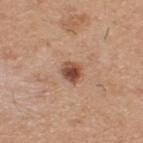{"automated_metrics": {"border_irregularity_0_10": 1.5, "color_variation_0_10": 5.0, "peripheral_color_asymmetry": 1.5, "nevus_likeness_0_100": 90}, "patient": {"sex": "male", "age_approx": 60}, "image": {"source": "total-body photography crop", "field_of_view_mm": 15}, "site": "upper back"}